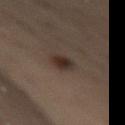Q: How was this image acquired?
A: ~15 mm tile from a whole-body skin photo
Q: Who is the patient?
A: male, aged 53 to 57
Q: Lesion location?
A: the right thigh